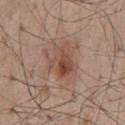Acquisition and patient details:
From the mid back. The patient is a male approximately 55 years of age. Cropped from a total-body skin-imaging series; the visible field is about 15 mm. About 4 mm across.